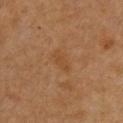Impression: The lesion was photographed on a routine skin check and not biopsied; there is no pathology result. Image and clinical context: The lesion is located on the front of the torso. Imaged with cross-polarized lighting. About 3.5 mm across. A female patient approximately 40 years of age. Cropped from a total-body skin-imaging series; the visible field is about 15 mm.